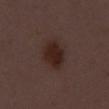Impression: The lesion was tiled from a total-body skin photograph and was not biopsied. Context: Cropped from a total-body skin-imaging series; the visible field is about 15 mm. The patient is a male in their 50s.